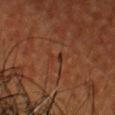| feature | finding |
|---|---|
| diameter | ≈2.5 mm |
| acquisition | total-body-photography crop, ~15 mm field of view |
| lighting | cross-polarized |
| site | the head or neck |
| subject | male, aged 48–52 |
| automated lesion analysis | a mean CIELAB color near L≈25 a*≈19 b*≈24, about 4 CIELAB-L* units darker than the surrounding skin, and a lesion-to-skin contrast of about 5 (normalized; higher = more distinct); a nevus-likeness score of about 0/100 |